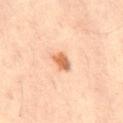notes=imaged on a skin check; not biopsied | image=total-body-photography crop, ~15 mm field of view | illumination=cross-polarized | patient=male, aged 48 to 52 | diameter=~3 mm (longest diameter) | automated lesion analysis=an area of roughly 4 mm², an eccentricity of roughly 0.8, and two-axis asymmetry of about 0.25; border irregularity of about 2 on a 0–10 scale; a classifier nevus-likeness of about 95/100 and a lesion-detection confidence of about 100/100 | anatomic site=the back.